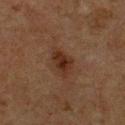Background: The lesion's longest dimension is about 4 mm. This is a cross-polarized tile. A male patient about 75 years old. Located on the chest. A 15 mm close-up tile from a total-body photography series done for melanoma screening. Automated tile analysis of the lesion measured a nevus-likeness score of about 70/100 and a detector confidence of about 100 out of 100 that the crop contains a lesion.